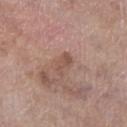Clinical impression: Captured during whole-body skin photography for melanoma surveillance; the lesion was not biopsied. Clinical summary: A lesion tile, about 15 mm wide, cut from a 3D total-body photograph. From the right lower leg. The lesion's longest dimension is about 3 mm. The patient is a female roughly 85 years of age or older.